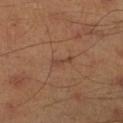biopsy status: imaged on a skin check; not biopsied | lesion size: ~2.5 mm (longest diameter) | tile lighting: cross-polarized illumination | image source: ~15 mm crop, total-body skin-cancer survey | image-analysis metrics: an area of roughly 2 mm², an eccentricity of roughly 0.95, and a symmetry-axis asymmetry near 0.35; a color-variation rating of about 0/10 and a peripheral color-asymmetry measure near 0 | patient: male, approximately 45 years of age | location: the right lower leg.